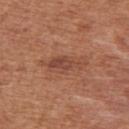Q: What kind of image is this?
A: 15 mm crop, total-body photography
Q: How large is the lesion?
A: ~5.5 mm (longest diameter)
Q: Patient demographics?
A: female, aged approximately 40
Q: Lesion location?
A: the upper back
Q: What lighting was used for the tile?
A: white-light